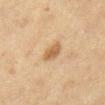notes: imaged on a skin check; not biopsied | illumination: cross-polarized illumination | subject: female, roughly 40 years of age | imaging modality: ~15 mm crop, total-body skin-cancer survey | automated lesion analysis: a footprint of about 5 mm², an outline eccentricity of about 0.55 (0 = round, 1 = elongated), and two-axis asymmetry of about 0.2; an average lesion color of about L≈52 a*≈16 b*≈34 (CIELAB), about 10 CIELAB-L* units darker than the surrounding skin, and a lesion-to-skin contrast of about 7.5 (normalized; higher = more distinct); a color-variation rating of about 2/10 and radial color variation of about 0.5 | site: the right lower leg | lesion diameter: about 2.5 mm.